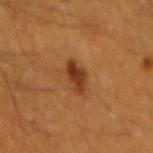Clinical impression:
The lesion was photographed on a routine skin check and not biopsied; there is no pathology result.
Acquisition and patient details:
A lesion tile, about 15 mm wide, cut from a 3D total-body photograph. Located on the mid back. A male subject about 60 years old.